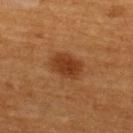Recorded during total-body skin imaging; not selected for excision or biopsy. The tile uses cross-polarized illumination. A male subject aged around 60. Located on the upper back. A lesion tile, about 15 mm wide, cut from a 3D total-body photograph. About 3.5 mm across.